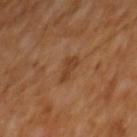Image and clinical context:
This is a cross-polarized tile. About 3 mm across. The total-body-photography lesion software estimated an automated nevus-likeness rating near 0 out of 100 and a lesion-detection confidence of about 100/100. A male subject, in their mid- to late 60s. A 15 mm close-up tile from a total-body photography series done for melanoma screening. The lesion is located on the mid back.